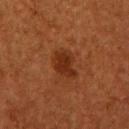notes — no biopsy performed (imaged during a skin exam); site — the arm; tile lighting — cross-polarized; patient — female, approximately 50 years of age; image — 15 mm crop, total-body photography; lesion size — ~4 mm (longest diameter); TBP lesion metrics — a footprint of about 7.5 mm² and an outline eccentricity of about 0.7 (0 = round, 1 = elongated).